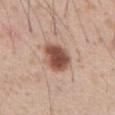On the abdomen.
Automated tile analysis of the lesion measured a normalized lesion–skin contrast near 11. It also reported a border-irregularity index near 1.5/10, internal color variation of about 4.5 on a 0–10 scale, and a peripheral color-asymmetry measure near 1.5.
A male patient aged around 55.
This image is a 15 mm lesion crop taken from a total-body photograph.